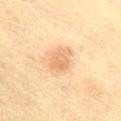A 15 mm close-up tile from a total-body photography series done for melanoma screening.
This is a cross-polarized tile.
The lesion is located on the lower back.
A female subject approximately 40 years of age.